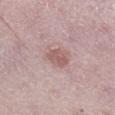The lesion was tiled from a total-body skin photograph and was not biopsied. On the right lower leg. A male subject, in their 50s. A 15 mm close-up tile from a total-body photography series done for melanoma screening. This is a white-light tile. The recorded lesion diameter is about 3 mm.